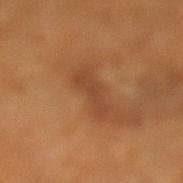Recorded during total-body skin imaging; not selected for excision or biopsy. The patient is a male aged 63–67. On the left lower leg. Measured at roughly 5 mm in maximum diameter. The lesion-visualizer software estimated a mean CIELAB color near L≈43 a*≈22 b*≈34, a lesion–skin lightness drop of about 7, and a normalized border contrast of about 6. A 15 mm crop from a total-body photograph taken for skin-cancer surveillance.